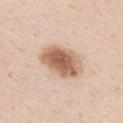follow-up=catalogued during a skin exam; not biopsied | anatomic site=the right upper arm | illumination=white-light illumination | patient=male, aged 28–32 | image source=~15 mm tile from a whole-body skin photo.